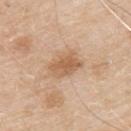<tbp_lesion>
  <biopsy_status>not biopsied; imaged during a skin examination</biopsy_status>
  <automated_metrics>
    <area_mm2_approx>8.5</area_mm2_approx>
    <eccentricity>0.75</eccentricity>
    <shape_asymmetry>0.2</shape_asymmetry>
    <cielab_L>60</cielab_L>
    <cielab_a>19</cielab_a>
    <cielab_b>35</cielab_b>
    <vs_skin_darker_L>10.0</vs_skin_darker_L>
    <vs_skin_contrast_norm>7.0</vs_skin_contrast_norm>
  </automated_metrics>
  <image>
    <source>total-body photography crop</source>
    <field_of_view_mm>15</field_of_view_mm>
  </image>
  <site>upper back</site>
  <lesion_size>
    <long_diameter_mm_approx>4.0</long_diameter_mm_approx>
  </lesion_size>
  <patient>
    <sex>male</sex>
    <age_approx>80</age_approx>
  </patient>
  <lighting>white-light</lighting>
</tbp_lesion>